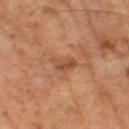Clinical impression: The lesion was tiled from a total-body skin photograph and was not biopsied. Context: Approximately 3 mm at its widest. A male patient aged 63 to 67. A region of skin cropped from a whole-body photographic capture, roughly 15 mm wide. Automated image analysis of the tile measured a lesion area of about 5.5 mm², an outline eccentricity of about 0.5 (0 = round, 1 = elongated), and a symmetry-axis asymmetry near 0.35. It also reported a border-irregularity rating of about 3.5/10, a color-variation rating of about 4.5/10, and radial color variation of about 2. The analysis additionally found an automated nevus-likeness rating near 0 out of 100. The tile uses cross-polarized illumination.